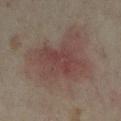Captured during whole-body skin photography for melanoma surveillance; the lesion was not biopsied.
Automated tile analysis of the lesion measured a mean CIELAB color near L≈42 a*≈15 b*≈20, about 7 CIELAB-L* units darker than the surrounding skin, and a normalized border contrast of about 5.5. The software also gave a border-irregularity index near 3.5/10, a color-variation rating of about 5/10, and a peripheral color-asymmetry measure near 1.5. And it measured a classifier nevus-likeness of about 15/100.
Cropped from a whole-body photographic skin survey; the tile spans about 15 mm.
A female patient, approximately 35 years of age.
This is a cross-polarized tile.
About 10 mm across.
The lesion is located on the left lower leg.